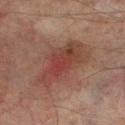follow-up: total-body-photography surveillance lesion; no biopsy
subject: male, aged approximately 75
body site: the left lower leg
lesion size: ~7 mm (longest diameter)
automated lesion analysis: a mean CIELAB color near L≈35 a*≈20 b*≈23, about 7 CIELAB-L* units darker than the surrounding skin, and a lesion-to-skin contrast of about 7 (normalized; higher = more distinct); a border-irregularity rating of about 4.5/10, a within-lesion color-variation index near 5.5/10, and radial color variation of about 2; a classifier nevus-likeness of about 35/100
illumination: cross-polarized illumination
acquisition: total-body-photography crop, ~15 mm field of view